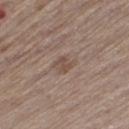The lesion was photographed on a routine skin check and not biopsied; there is no pathology result. Located on the leg. A male subject aged 63 to 67. A 15 mm close-up extracted from a 3D total-body photography capture.